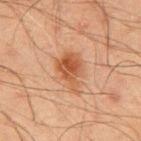Clinical impression: The lesion was tiled from a total-body skin photograph and was not biopsied. Clinical summary: This image is a 15 mm lesion crop taken from a total-body photograph. A male subject, roughly 45 years of age. Automated tile analysis of the lesion measured an area of roughly 11 mm², an eccentricity of roughly 0.75, and a shape-asymmetry score of about 0.3 (0 = symmetric). The analysis additionally found about 9 CIELAB-L* units darker than the surrounding skin. The analysis additionally found a border-irregularity index near 4/10, a color-variation rating of about 5/10, and a peripheral color-asymmetry measure near 1.5. It also reported a classifier nevus-likeness of about 90/100 and a lesion-detection confidence of about 100/100. The tile uses cross-polarized illumination. On the upper back. The recorded lesion diameter is about 5 mm.